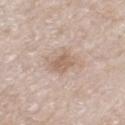Impression:
The lesion was photographed on a routine skin check and not biopsied; there is no pathology result.
Background:
A female patient approximately 75 years of age. A 15 mm close-up extracted from a 3D total-body photography capture. The recorded lesion diameter is about 4 mm. From the leg. Automated tile analysis of the lesion measured about 8 CIELAB-L* units darker than the surrounding skin and a normalized border contrast of about 5.5. Imaged with white-light lighting.